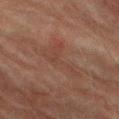The lesion is located on the left thigh.
The lesion's longest dimension is about 5.5 mm.
The patient is a male roughly 75 years of age.
Cropped from a total-body skin-imaging series; the visible field is about 15 mm.
Captured under cross-polarized illumination.